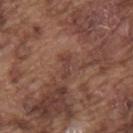Recorded during total-body skin imaging; not selected for excision or biopsy.
From the upper back.
Automated tile analysis of the lesion measured an average lesion color of about L≈40 a*≈21 b*≈24 (CIELAB). The analysis additionally found border irregularity of about 5 on a 0–10 scale, a color-variation rating of about 0/10, and a peripheral color-asymmetry measure near 0.
Captured under white-light illumination.
A male subject, about 75 years old.
A 15 mm crop from a total-body photograph taken for skin-cancer surveillance.
Approximately 2.5 mm at its widest.